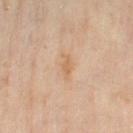Clinical impression: Recorded during total-body skin imaging; not selected for excision or biopsy. Background: On the left thigh. A female patient aged around 60. A 15 mm close-up extracted from a 3D total-body photography capture. About 3 mm across. Imaged with cross-polarized lighting.